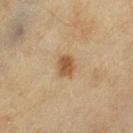Captured during whole-body skin photography for melanoma surveillance; the lesion was not biopsied.
The patient is a female aged 53 to 57.
On the left thigh.
Approximately 2.5 mm at its widest.
Cropped from a total-body skin-imaging series; the visible field is about 15 mm.
An algorithmic analysis of the crop reported a lesion color around L≈47 a*≈16 b*≈32 in CIELAB, about 10 CIELAB-L* units darker than the surrounding skin, and a lesion-to-skin contrast of about 8 (normalized; higher = more distinct).